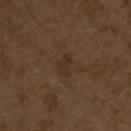Q: Is there a histopathology result?
A: total-body-photography surveillance lesion; no biopsy
Q: How was the tile lit?
A: cross-polarized
Q: Lesion size?
A: ≈2.5 mm
Q: What is the imaging modality?
A: 15 mm crop, total-body photography
Q: What is the anatomic site?
A: the front of the torso
Q: Patient demographics?
A: male, aged 58 to 62
Q: What did automated image analysis measure?
A: a footprint of about 3 mm² and an outline eccentricity of about 0.85 (0 = round, 1 = elongated); a peripheral color-asymmetry measure near 0.5